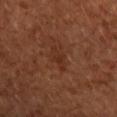Assessment: No biopsy was performed on this lesion — it was imaged during a full skin examination and was not determined to be concerning. Background: A lesion tile, about 15 mm wide, cut from a 3D total-body photograph. The lesion is located on the right forearm. A female subject aged around 65.